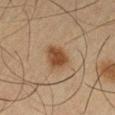Background: A male patient in their mid-60s. This is a cross-polarized tile. Automated tile analysis of the lesion measured an area of roughly 6.5 mm² and a shape-asymmetry score of about 0.2 (0 = symmetric). It also reported a lesion color around L≈37 a*≈17 b*≈29 in CIELAB, about 10 CIELAB-L* units darker than the surrounding skin, and a lesion-to-skin contrast of about 10 (normalized; higher = more distinct). The analysis additionally found an automated nevus-likeness rating near 100 out of 100 and a lesion-detection confidence of about 100/100. Cropped from a whole-body photographic skin survey; the tile spans about 15 mm. Longest diameter approximately 3 mm. Located on the leg.